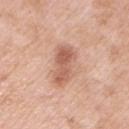biopsy status: no biopsy performed (imaged during a skin exam) | image: ~15 mm crop, total-body skin-cancer survey | automated lesion analysis: an area of roughly 9.5 mm², an outline eccentricity of about 0.9 (0 = round, 1 = elongated), and two-axis asymmetry of about 0.2; a mean CIELAB color near L≈60 a*≈23 b*≈30, roughly 12 lightness units darker than nearby skin, and a normalized lesion–skin contrast near 7.5; a nevus-likeness score of about 20/100 and a detector confidence of about 100 out of 100 that the crop contains a lesion | tile lighting: white-light | anatomic site: the left upper arm | subject: male, aged 53–57 | lesion size: about 5 mm.